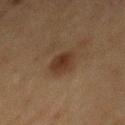The lesion was photographed on a routine skin check and not biopsied; there is no pathology result. A male patient, aged around 75. Approximately 3.5 mm at its widest. From the mid back. The tile uses cross-polarized illumination. A 15 mm close-up tile from a total-body photography series done for melanoma screening.